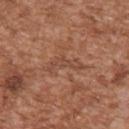The lesion was tiled from a total-body skin photograph and was not biopsied. Imaged with white-light lighting. Longest diameter approximately 4.5 mm. A male subject aged 43 to 47. On the arm. Cropped from a total-body skin-imaging series; the visible field is about 15 mm.